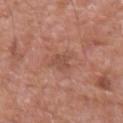  biopsy_status: not biopsied; imaged during a skin examination
  image:
    source: total-body photography crop
    field_of_view_mm: 15
  lesion_size:
    long_diameter_mm_approx: 3.0
  lighting: white-light
  site: right upper arm
  patient:
    sex: male
    age_approx: 80